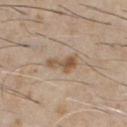Case summary:
• notes · imaged on a skin check; not biopsied
• patient · male, in their 60s
• site · the chest
• image · ~15 mm crop, total-body skin-cancer survey